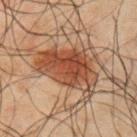biopsy_status: not biopsied; imaged during a skin examination
site: right upper arm
image:
  source: total-body photography crop
  field_of_view_mm: 15
automated_metrics:
  cielab_L: 35
  cielab_a: 19
  cielab_b: 26
  vs_skin_contrast_norm: 9.5
  color_variation_0_10: 5.0
  peripheral_color_asymmetry: 1.5
  nevus_likeness_0_100: 85
patient:
  sex: male
  age_approx: 50